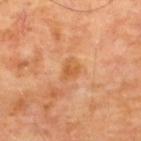Captured during whole-body skin photography for melanoma surveillance; the lesion was not biopsied. Imaged with cross-polarized lighting. On the upper back. Automated tile analysis of the lesion measured a mean CIELAB color near L≈56 a*≈25 b*≈41 and a normalized lesion–skin contrast near 6.5. The software also gave a within-lesion color-variation index near 2.5/10 and radial color variation of about 1. And it measured a classifier nevus-likeness of about 0/100. The subject is a male approximately 65 years of age. A 15 mm crop from a total-body photograph taken for skin-cancer surveillance. About 3 mm across.